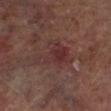body site: the left lower leg | lesion size: ~5 mm (longest diameter) | patient: male, about 65 years old | image-analysis metrics: a shape eccentricity near 0.6; an average lesion color of about L≈31 a*≈21 b*≈19 (CIELAB), a lesion–skin lightness drop of about 5, and a normalized border contrast of about 5.5; a border-irregularity rating of about 6/10 and a within-lesion color-variation index near 4/10; a classifier nevus-likeness of about 0/100 and a lesion-detection confidence of about 100/100 | lighting: cross-polarized illumination | image: 15 mm crop, total-body photography.